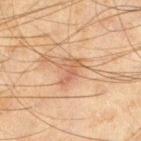Assessment: Imaged during a routine full-body skin examination; the lesion was not biopsied and no histopathology is available. Context: A 15 mm close-up extracted from a 3D total-body photography capture. Located on the left thigh. The subject is a male aged 43 to 47.